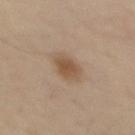{"image": {"source": "total-body photography crop", "field_of_view_mm": 15}, "patient": {"sex": "male", "age_approx": 55}, "lighting": "cross-polarized", "site": "abdomen", "automated_metrics": {"area_mm2_approx": 7.5, "eccentricity": 0.35, "shape_asymmetry": 0.15, "vs_skin_darker_L": 9.0, "vs_skin_contrast_norm": 7.0, "border_irregularity_0_10": 1.5, "peripheral_color_asymmetry": 1.0}}